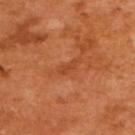notes=imaged on a skin check; not biopsied